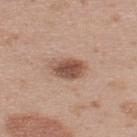notes: no biopsy performed (imaged during a skin exam) | size: ~4 mm (longest diameter) | patient: male, aged around 35 | imaging modality: total-body-photography crop, ~15 mm field of view | anatomic site: the upper back.